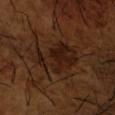Image and clinical context: The patient is a male about 65 years old. Approximately 6 mm at its widest. Cropped from a total-body skin-imaging series; the visible field is about 15 mm. From the left forearm. Captured under cross-polarized illumination.